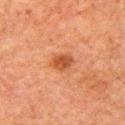biopsy_status: not biopsied; imaged during a skin examination
automated_metrics:
  area_mm2_approx: 5.0
  eccentricity: 0.65
  shape_asymmetry: 0.2
  border_irregularity_0_10: 1.5
  peripheral_color_asymmetry: 1.0
  lesion_detection_confidence_0_100: 100
lighting: cross-polarized
patient:
  sex: male
  age_approx: 60
image:
  source: total-body photography crop
  field_of_view_mm: 15
lesion_size:
  long_diameter_mm_approx: 3.0
site: right upper arm